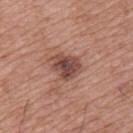subject: male, approximately 65 years of age | tile lighting: white-light illumination | lesion diameter: ~4 mm (longest diameter) | site: the upper back | imaging modality: 15 mm crop, total-body photography.